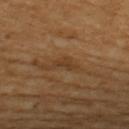Q: Was this lesion biopsied?
A: no biopsy performed (imaged during a skin exam)
Q: Patient demographics?
A: female, in their 50s
Q: How large is the lesion?
A: about 2.5 mm
Q: What kind of image is this?
A: total-body-photography crop, ~15 mm field of view
Q: What did automated image analysis measure?
A: a lesion area of about 2 mm² and a symmetry-axis asymmetry near 0.45
Q: What lighting was used for the tile?
A: cross-polarized
Q: Where on the body is the lesion?
A: the upper back